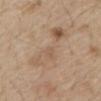Notes:
• biopsy status · imaged on a skin check; not biopsied
• lesion size · about 8.5 mm
• automated lesion analysis · a shape eccentricity near 0.95 and two-axis asymmetry of about 0.5; a normalized border contrast of about 4.5; a border-irregularity index near 7/10 and a within-lesion color-variation index near 6/10; an automated nevus-likeness rating near 0 out of 100
• anatomic site · the abdomen
• imaging modality · ~15 mm tile from a whole-body skin photo
• subject · male, roughly 70 years of age
• lighting · white-light illumination